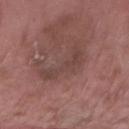<record>
<biopsy_status>not biopsied; imaged during a skin examination</biopsy_status>
<image>
  <source>total-body photography crop</source>
  <field_of_view_mm>15</field_of_view_mm>
</image>
<patient>
  <sex>male</sex>
  <age_approx>55</age_approx>
</patient>
<site>right forearm</site>
</record>